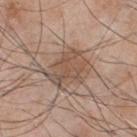Notes:
* biopsy status — total-body-photography surveillance lesion; no biopsy
* body site — the chest
* patient — male, approximately 50 years of age
* image source — ~15 mm crop, total-body skin-cancer survey
* lighting — white-light illumination
* size — about 5 mm